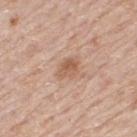Clinical summary: A male patient in their 80s. Located on the upper back. This image is a 15 mm lesion crop taken from a total-body photograph. Longest diameter approximately 3 mm. This is a white-light tile.